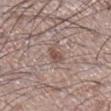<lesion>
<biopsy_status>not biopsied; imaged during a skin examination</biopsy_status>
<lighting>white-light</lighting>
<automated_metrics>
  <area_mm2_approx>3.5</area_mm2_approx>
  <eccentricity>0.9</eccentricity>
  <color_variation_0_10>2.0</color_variation_0_10>
  <peripheral_color_asymmetry>0.5</peripheral_color_asymmetry>
  <nevus_likeness_0_100>5</nevus_likeness_0_100>
  <lesion_detection_confidence_0_100>100</lesion_detection_confidence_0_100>
</automated_metrics>
<site>right lower leg</site>
<patient>
  <sex>male</sex>
  <age_approx>60</age_approx>
</patient>
<image>
  <source>total-body photography crop</source>
  <field_of_view_mm>15</field_of_view_mm>
</image>
</lesion>